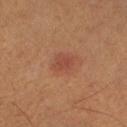Impression:
The lesion was tiled from a total-body skin photograph and was not biopsied.
Acquisition and patient details:
On the right forearm. Cropped from a whole-body photographic skin survey; the tile spans about 15 mm. The patient is a male aged around 60. This is a cross-polarized tile.